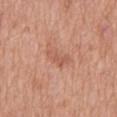Captured during whole-body skin photography for melanoma surveillance; the lesion was not biopsied.
On the mid back.
A 15 mm close-up tile from a total-body photography series done for melanoma screening.
This is a white-light tile.
The lesion's longest dimension is about 3 mm.
A male patient aged 68 to 72.
Automated image analysis of the tile measured a normalized border contrast of about 5.